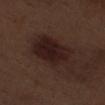Clinical impression: Recorded during total-body skin imaging; not selected for excision or biopsy. Context: A male patient aged around 70. This image is a 15 mm lesion crop taken from a total-body photograph. Located on the right thigh. The total-body-photography lesion software estimated a footprint of about 21 mm² and an eccentricity of roughly 0.8. The recorded lesion diameter is about 6.5 mm.